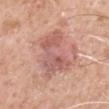{
  "site": "right upper arm",
  "patient": {
    "sex": "male",
    "age_approx": 60
  },
  "image": {
    "source": "total-body photography crop",
    "field_of_view_mm": 15
  }
}